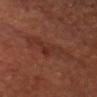Q: Was this lesion biopsied?
A: catalogued during a skin exam; not biopsied
Q: What is the imaging modality?
A: 15 mm crop, total-body photography
Q: What is the anatomic site?
A: the head or neck
Q: What lighting was used for the tile?
A: cross-polarized
Q: Patient demographics?
A: male, approximately 65 years of age